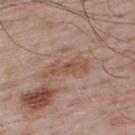No biopsy was performed on this lesion — it was imaged during a full skin examination and was not determined to be concerning.
Imaged with white-light lighting.
Longest diameter approximately 5 mm.
Cropped from a total-body skin-imaging series; the visible field is about 15 mm.
Automated image analysis of the tile measured a lesion–skin lightness drop of about 8 and a normalized lesion–skin contrast near 6.5. It also reported a classifier nevus-likeness of about 0/100 and a detector confidence of about 90 out of 100 that the crop contains a lesion.
A male patient aged 63 to 67.
From the upper back.